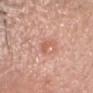notes: no biopsy performed (imaged during a skin exam)
image source: 15 mm crop, total-body photography
illumination: white-light
location: the head or neck
automated lesion analysis: a mean CIELAB color near L≈60 a*≈26 b*≈31, roughly 8 lightness units darker than nearby skin, and a normalized lesion–skin contrast near 5.5; a lesion-detection confidence of about 100/100
patient: male, aged 43 to 47